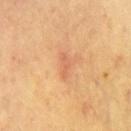Assessment: No biopsy was performed on this lesion — it was imaged during a full skin examination and was not determined to be concerning. Image and clinical context: On the chest. Automated image analysis of the tile measured an area of roughly 2.5 mm² and a shape eccentricity near 0.95. The software also gave a normalized border contrast of about 5. The software also gave border irregularity of about 6 on a 0–10 scale, a color-variation rating of about 0/10, and peripheral color asymmetry of about 0. A region of skin cropped from a whole-body photographic capture, roughly 15 mm wide. A male subject aged around 70. The lesion's longest dimension is about 3 mm.